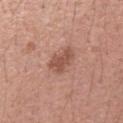{
  "biopsy_status": "not biopsied; imaged during a skin examination",
  "automated_metrics": {
    "area_mm2_approx": 6.0,
    "eccentricity": 0.75,
    "shape_asymmetry": 0.35,
    "border_irregularity_0_10": 3.5,
    "color_variation_0_10": 1.5,
    "nevus_likeness_0_100": 35,
    "lesion_detection_confidence_0_100": 100
  },
  "patient": {
    "sex": "female",
    "age_approx": 35
  },
  "image": {
    "source": "total-body photography crop",
    "field_of_view_mm": 15
  },
  "lesion_size": {
    "long_diameter_mm_approx": 3.5
  },
  "lighting": "white-light",
  "site": "left forearm"
}